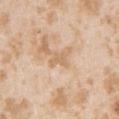Q: Is there a histopathology result?
A: no biopsy performed (imaged during a skin exam)
Q: How was this image acquired?
A: total-body-photography crop, ~15 mm field of view
Q: What lighting was used for the tile?
A: white-light illumination
Q: Lesion location?
A: the left upper arm
Q: Patient demographics?
A: female, roughly 25 years of age
Q: What did automated image analysis measure?
A: an average lesion color of about L≈66 a*≈17 b*≈36 (CIELAB), roughly 7 lightness units darker than nearby skin, and a lesion-to-skin contrast of about 5.5 (normalized; higher = more distinct); border irregularity of about 4.5 on a 0–10 scale, a color-variation rating of about 1/10, and a peripheral color-asymmetry measure near 0.5; a detector confidence of about 100 out of 100 that the crop contains a lesion
Q: What is the lesion's diameter?
A: ~2.5 mm (longest diameter)